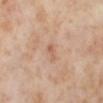No biopsy was performed on this lesion — it was imaged during a full skin examination and was not determined to be concerning. A female patient, approximately 55 years of age. The lesion is on the left lower leg. Automated image analysis of the tile measured a footprint of about 2 mm², an eccentricity of roughly 0.95, and a symmetry-axis asymmetry near 0.45. And it measured internal color variation of about 0 on a 0–10 scale and radial color variation of about 0. Cropped from a whole-body photographic skin survey; the tile spans about 15 mm. Captured under cross-polarized illumination.